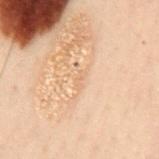Findings:
- notes: imaged on a skin check; not biopsied
- site: the mid back
- image source: total-body-photography crop, ~15 mm field of view
- patient: male, about 50 years old
- illumination: cross-polarized illumination
- diameter: about 2 mm